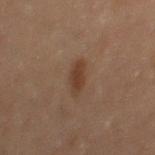<record>
<biopsy_status>not biopsied; imaged during a skin examination</biopsy_status>
</record>